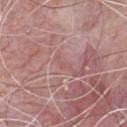{"biopsy_status": "not biopsied; imaged during a skin examination", "patient": {"sex": "male", "age_approx": 70}, "site": "chest", "lighting": "white-light", "lesion_size": {"long_diameter_mm_approx": 2.0}, "automated_metrics": {"area_mm2_approx": 1.5, "eccentricity": 0.9, "shape_asymmetry": 0.6, "vs_skin_contrast_norm": 4.0}, "image": {"source": "total-body photography crop", "field_of_view_mm": 15}}